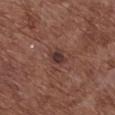follow-up: no biopsy performed (imaged during a skin exam) | body site: the chest | subject: female, aged 78–82 | image source: ~15 mm crop, total-body skin-cancer survey | diameter: ~2.5 mm (longest diameter) | tile lighting: white-light illumination | TBP lesion metrics: an area of roughly 4 mm², a shape eccentricity near 0.6, and two-axis asymmetry of about 0.2; border irregularity of about 2 on a 0–10 scale; a classifier nevus-likeness of about 90/100 and lesion-presence confidence of about 100/100.